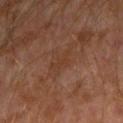notes: imaged on a skin check; not biopsied
tile lighting: cross-polarized illumination
imaging modality: ~15 mm crop, total-body skin-cancer survey
patient: male, aged 28 to 32
location: the left arm
lesion diameter: ≈3.5 mm